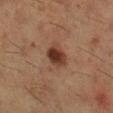Q: Is there a histopathology result?
A: catalogued during a skin exam; not biopsied
Q: How large is the lesion?
A: about 3 mm
Q: Patient demographics?
A: female, in their 50s
Q: How was the tile lit?
A: cross-polarized
Q: What is the imaging modality?
A: ~15 mm crop, total-body skin-cancer survey
Q: What did automated image analysis measure?
A: a footprint of about 6.5 mm², a shape eccentricity near 0.6, and a shape-asymmetry score of about 0.2 (0 = symmetric); an average lesion color of about L≈29 a*≈18 b*≈23 (CIELAB), roughly 11 lightness units darker than nearby skin, and a lesion-to-skin contrast of about 10.5 (normalized; higher = more distinct); a border-irregularity index near 1.5/10 and internal color variation of about 4.5 on a 0–10 scale; a classifier nevus-likeness of about 95/100 and a detector confidence of about 100 out of 100 that the crop contains a lesion
Q: What is the anatomic site?
A: the right lower leg